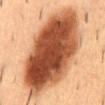No biopsy was performed on this lesion — it was imaged during a full skin examination and was not determined to be concerning. The lesion is on the mid back. Longest diameter approximately 13.5 mm. The lesion-visualizer software estimated two-axis asymmetry of about 0.1. It also reported a border-irregularity index near 2/10 and a peripheral color-asymmetry measure near 3. It also reported an automated nevus-likeness rating near 100 out of 100 and lesion-presence confidence of about 100/100. Cropped from a total-body skin-imaging series; the visible field is about 15 mm. This is a cross-polarized tile. A male subject in their mid-50s.